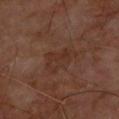Part of a total-body skin-imaging series; this lesion was reviewed on a skin check and was not flagged for biopsy. The lesion-visualizer software estimated a lesion area of about 9 mm² and an eccentricity of roughly 0.8. The software also gave an average lesion color of about L≈31 a*≈19 b*≈24 (CIELAB), a lesion–skin lightness drop of about 4, and a normalized border contrast of about 5. And it measured a border-irregularity rating of about 3.5/10 and a color-variation rating of about 3/10. It also reported a nevus-likeness score of about 0/100 and a detector confidence of about 100 out of 100 that the crop contains a lesion. Cropped from a whole-body photographic skin survey; the tile spans about 15 mm. Imaged with cross-polarized lighting. The recorded lesion diameter is about 4.5 mm. The lesion is located on the upper back. The subject is a male aged 68–72.